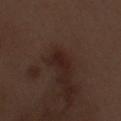Q: What lighting was used for the tile?
A: white-light
Q: What kind of image is this?
A: 15 mm crop, total-body photography
Q: What is the anatomic site?
A: the left forearm
Q: What did automated image analysis measure?
A: a lesion area of about 2.5 mm² and two-axis asymmetry of about 0.3
Q: Who is the patient?
A: male, roughly 70 years of age